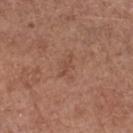Assessment: Imaged during a routine full-body skin examination; the lesion was not biopsied and no histopathology is available. Background: A 15 mm close-up extracted from a 3D total-body photography capture. From the left forearm. A female subject, in their mid-60s. Measured at roughly 3 mm in maximum diameter.